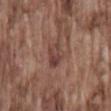<record>
  <biopsy_status>not biopsied; imaged during a skin examination</biopsy_status>
  <image>
    <source>total-body photography crop</source>
    <field_of_view_mm>15</field_of_view_mm>
  </image>
  <patient>
    <sex>male</sex>
    <age_approx>75</age_approx>
  </patient>
  <site>lower back</site>
  <lesion_size>
    <long_diameter_mm_approx>3.0</long_diameter_mm_approx>
  </lesion_size>
  <lighting>white-light</lighting>
</record>